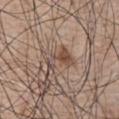Assessment: Part of a total-body skin-imaging series; this lesion was reviewed on a skin check and was not flagged for biopsy. Context: Measured at roughly 3.5 mm in maximum diameter. The lesion is on the abdomen. The subject is a male aged 73–77. Imaged with white-light lighting. This image is a 15 mm lesion crop taken from a total-body photograph.